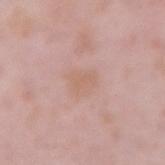Clinical impression: Recorded during total-body skin imaging; not selected for excision or biopsy. Background: A male patient aged around 55. This image is a 15 mm lesion crop taken from a total-body photograph. On the right upper arm. The total-body-photography lesion software estimated a mean CIELAB color near L≈63 a*≈19 b*≈27, roughly 5 lightness units darker than nearby skin, and a normalized border contrast of about 4.5. This is a white-light tile. The lesion's longest dimension is about 3 mm.